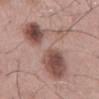biopsy status: imaged on a skin check; not biopsied | image: total-body-photography crop, ~15 mm field of view | image-analysis metrics: a footprint of about 43 mm², an outline eccentricity of about 0.9 (0 = round, 1 = elongated), and a symmetry-axis asymmetry near 0.4; a lesion color around L≈54 a*≈18 b*≈23 in CIELAB and a normalized lesion–skin contrast near 7 | subject: male, in their mid-50s | location: the mid back | illumination: white-light | diameter: ≈11.5 mm.